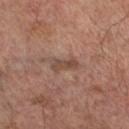| feature | finding |
|---|---|
| workup | catalogued during a skin exam; not biopsied |
| site | the right lower leg |
| tile lighting | cross-polarized |
| automated metrics | a nevus-likeness score of about 0/100 and a detector confidence of about 95 out of 100 that the crop contains a lesion |
| size | about 3 mm |
| image source | 15 mm crop, total-body photography |
| patient | male, roughly 65 years of age |